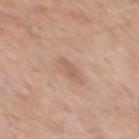Notes:
- biopsy status: total-body-photography surveillance lesion; no biopsy
- image source: total-body-photography crop, ~15 mm field of view
- TBP lesion metrics: an outline eccentricity of about 0.9 (0 = round, 1 = elongated); an average lesion color of about L≈59 a*≈20 b*≈28 (CIELAB), a lesion–skin lightness drop of about 8, and a lesion-to-skin contrast of about 5 (normalized; higher = more distinct)
- subject: male, roughly 60 years of age
- tile lighting: white-light illumination
- body site: the mid back
- lesion size: ~2.5 mm (longest diameter)